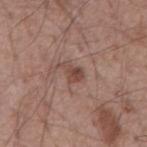Impression: No biopsy was performed on this lesion — it was imaged during a full skin examination and was not determined to be concerning. Background: A male subject, about 55 years old. Captured under white-light illumination. From the mid back. A 15 mm crop from a total-body photograph taken for skin-cancer surveillance. The lesion-visualizer software estimated border irregularity of about 5 on a 0–10 scale, a color-variation rating of about 2/10, and radial color variation of about 0.5.